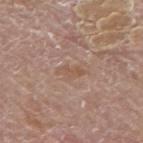biopsy_status: not biopsied; imaged during a skin examination
site: mid back
image:
  source: total-body photography crop
  field_of_view_mm: 15
lighting: white-light
patient:
  sex: male
  age_approx: 80
lesion_size:
  long_diameter_mm_approx: 3.0